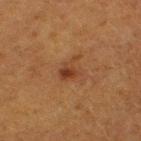Captured during whole-body skin photography for melanoma surveillance; the lesion was not biopsied. This image is a 15 mm lesion crop taken from a total-body photograph. Imaged with cross-polarized lighting. Located on the left thigh. The subject is a female aged 48 to 52. An algorithmic analysis of the crop reported an area of roughly 4.5 mm², a shape eccentricity near 0.65, and a shape-asymmetry score of about 0.45 (0 = symmetric). The analysis additionally found a mean CIELAB color near L≈33 a*≈20 b*≈29, about 7 CIELAB-L* units darker than the surrounding skin, and a lesion-to-skin contrast of about 7 (normalized; higher = more distinct). And it measured border irregularity of about 5.5 on a 0–10 scale. The lesion's longest dimension is about 3 mm.